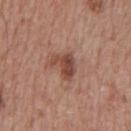Recorded during total-body skin imaging; not selected for excision or biopsy. A region of skin cropped from a whole-body photographic capture, roughly 15 mm wide. A male subject, aged approximately 65. This is a white-light tile. From the chest. Automated image analysis of the tile measured an outline eccentricity of about 0.75 (0 = round, 1 = elongated) and two-axis asymmetry of about 0.5. The software also gave a border-irregularity rating of about 5/10, a within-lesion color-variation index near 4/10, and peripheral color asymmetry of about 1.5.